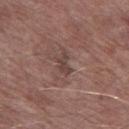Case summary:
- workup — catalogued during a skin exam; not biopsied
- lesion diameter — ≈3 mm
- automated lesion analysis — a border-irregularity index near 5/10, a color-variation rating of about 0.5/10, and peripheral color asymmetry of about 0; a detector confidence of about 80 out of 100 that the crop contains a lesion
- image source — 15 mm crop, total-body photography
- subject — male, aged around 75
- lighting — white-light
- anatomic site — the left thigh